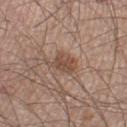No biopsy was performed on this lesion — it was imaged during a full skin examination and was not determined to be concerning.
An algorithmic analysis of the crop reported a footprint of about 6 mm² and a symmetry-axis asymmetry near 0.35. And it measured an average lesion color of about L≈48 a*≈18 b*≈27 (CIELAB) and a normalized lesion–skin contrast near 7.
On the right lower leg.
A 15 mm close-up tile from a total-body photography series done for melanoma screening.
A male patient roughly 60 years of age.